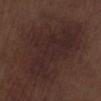Assessment: Recorded during total-body skin imaging; not selected for excision or biopsy. Background: Longest diameter approximately 11.5 mm. A 15 mm close-up tile from a total-body photography series done for melanoma screening. The patient is a male in their 70s. The lesion is located on the leg. Captured under white-light illumination.